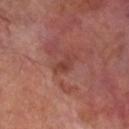Impression: Part of a total-body skin-imaging series; this lesion was reviewed on a skin check and was not flagged for biopsy. Background: The lesion is on the right lower leg. A 15 mm close-up tile from a total-body photography series done for melanoma screening. The subject is a male aged 68 to 72.